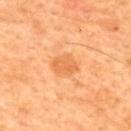follow-up = no biopsy performed (imaged during a skin exam)
image source = ~15 mm crop, total-body skin-cancer survey
body site = the upper back
subject = male, in their mid-40s
lighting = cross-polarized illumination
lesion diameter = about 3 mm